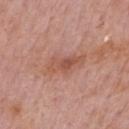The lesion was tiled from a total-body skin photograph and was not biopsied.
A male patient, approximately 75 years of age.
The lesion-visualizer software estimated a lesion area of about 9.5 mm², a shape eccentricity near 0.9, and a symmetry-axis asymmetry near 0.3. It also reported a mean CIELAB color near L≈54 a*≈24 b*≈29 and roughly 8 lightness units darker than nearby skin. The software also gave a border-irregularity index near 3.5/10, a within-lesion color-variation index near 4/10, and radial color variation of about 1.
A lesion tile, about 15 mm wide, cut from a 3D total-body photograph.
The lesion is located on the mid back.
Longest diameter approximately 5 mm.